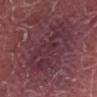Q: What are the patient's age and sex?
A: male, in their 40s
Q: How was this image acquired?
A: ~15 mm tile from a whole-body skin photo
Q: Where on the body is the lesion?
A: the abdomen